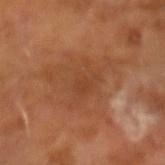<case>
<biopsy_status>not biopsied; imaged during a skin examination</biopsy_status>
<automated_metrics>
  <area_mm2_approx>30.0</area_mm2_approx>
  <eccentricity>0.55</eccentricity>
  <shape_asymmetry>0.4</shape_asymmetry>
</automated_metrics>
<image>
  <source>total-body photography crop</source>
  <field_of_view_mm>15</field_of_view_mm>
</image>
<lighting>cross-polarized</lighting>
<lesion_size>
  <long_diameter_mm_approx>8.5</long_diameter_mm_approx>
</lesion_size>
<patient>
  <sex>male</sex>
  <age_approx>65</age_approx>
</patient>
</case>